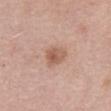Impression:
The lesion was photographed on a routine skin check and not biopsied; there is no pathology result.
Clinical summary:
The tile uses white-light illumination. A female subject, aged approximately 60. A 15 mm crop from a total-body photograph taken for skin-cancer surveillance. The lesion is located on the abdomen. Measured at roughly 3 mm in maximum diameter.